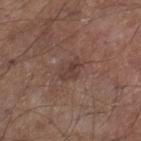acquisition: total-body-photography crop, ~15 mm field of view | diameter: about 3 mm | site: the leg | subject: male, about 55 years old | TBP lesion metrics: a shape eccentricity near 0.85 and a shape-asymmetry score of about 0.35 (0 = symmetric); roughly 7 lightness units darker than nearby skin and a lesion-to-skin contrast of about 6 (normalized; higher = more distinct); a within-lesion color-variation index near 3/10 and radial color variation of about 1 | illumination: white-light.